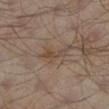The lesion was photographed on a routine skin check and not biopsied; there is no pathology result. A lesion tile, about 15 mm wide, cut from a 3D total-body photograph. A male subject, aged around 65. On the left lower leg. The recorded lesion diameter is about 3.5 mm. The total-body-photography lesion software estimated a symmetry-axis asymmetry near 0.35. It also reported a mean CIELAB color near L≈41 a*≈13 b*≈24, a lesion–skin lightness drop of about 6, and a normalized border contrast of about 6. And it measured a border-irregularity rating of about 4.5/10, internal color variation of about 1 on a 0–10 scale, and a peripheral color-asymmetry measure near 0.5. The tile uses cross-polarized illumination.